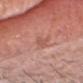workup: no biopsy performed (imaged during a skin exam)
image-analysis metrics: a footprint of about 2.5 mm² and two-axis asymmetry of about 0.25
subject: male, aged 58–62
tile lighting: white-light illumination
lesion diameter: ≈3 mm
image source: total-body-photography crop, ~15 mm field of view
location: the head or neck